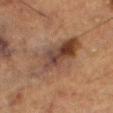Findings:
- image — 15 mm crop, total-body photography
- site — the leg
- tile lighting — cross-polarized
- patient — male, about 75 years old